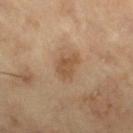workup = no biopsy performed (imaged during a skin exam)
patient = male, aged approximately 65
image = ~15 mm tile from a whole-body skin photo
lighting = cross-polarized illumination
automated metrics = a lesion area of about 6.5 mm², a shape eccentricity near 0.65, and two-axis asymmetry of about 0.35; an average lesion color of about L≈50 a*≈17 b*≈33 (CIELAB)
diameter = ~3.5 mm (longest diameter)